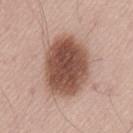This lesion was catalogued during total-body skin photography and was not selected for biopsy.
Located on the lower back.
A 15 mm close-up extracted from a 3D total-body photography capture.
The lesion's longest dimension is about 7 mm.
Imaged with white-light lighting.
The subject is a male about 55 years old.